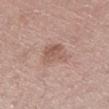This lesion was catalogued during total-body skin photography and was not selected for biopsy.